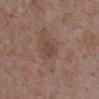* follow-up: no biopsy performed (imaged during a skin exam)
* site: the upper back
* imaging modality: 15 mm crop, total-body photography
* subject: female, roughly 35 years of age